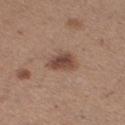Assessment: Imaged during a routine full-body skin examination; the lesion was not biopsied and no histopathology is available. Clinical summary: Located on the right thigh. The tile uses white-light illumination. A 15 mm close-up tile from a total-body photography series done for melanoma screening. Measured at roughly 3.5 mm in maximum diameter. A female subject in their 30s.